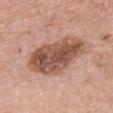Assessment:
This lesion was catalogued during total-body skin photography and was not selected for biopsy.
Context:
The lesion is located on the chest. Automated tile analysis of the lesion measured a lesion area of about 26 mm², an outline eccentricity of about 0.9 (0 = round, 1 = elongated), and a shape-asymmetry score of about 0.15 (0 = symmetric). It also reported a border-irregularity rating of about 2.5/10 and radial color variation of about 2.5. And it measured an automated nevus-likeness rating near 10 out of 100 and a lesion-detection confidence of about 100/100. A 15 mm crop from a total-body photograph taken for skin-cancer surveillance. Measured at roughly 8.5 mm in maximum diameter. A female subject roughly 60 years of age. Captured under white-light illumination.